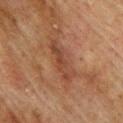Clinical impression:
Captured during whole-body skin photography for melanoma surveillance; the lesion was not biopsied.
Clinical summary:
Cropped from a total-body skin-imaging series; the visible field is about 15 mm. On the upper back. A male subject roughly 75 years of age.